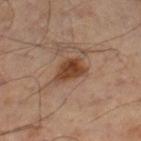Assessment:
Recorded during total-body skin imaging; not selected for excision or biopsy.
Image and clinical context:
About 4 mm across. Automated tile analysis of the lesion measured a border-irregularity rating of about 3.5/10, a color-variation rating of about 3.5/10, and radial color variation of about 1. It also reported an automated nevus-likeness rating near 90 out of 100 and lesion-presence confidence of about 100/100. This is a cross-polarized tile. The lesion is on the left leg. Cropped from a whole-body photographic skin survey; the tile spans about 15 mm. A male subject, aged approximately 50.